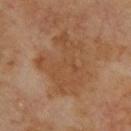This lesion was catalogued during total-body skin photography and was not selected for biopsy. Longest diameter approximately 7 mm. A male subject in their 70s. The tile uses cross-polarized illumination. This image is a 15 mm lesion crop taken from a total-body photograph. From the upper back. Automated tile analysis of the lesion measured a lesion area of about 28 mm². The analysis additionally found an average lesion color of about L≈47 a*≈20 b*≈33 (CIELAB), roughly 6 lightness units darker than nearby skin, and a normalized lesion–skin contrast near 5.5.